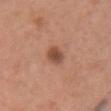A lesion tile, about 15 mm wide, cut from a 3D total-body photograph.
A female subject aged 33 to 37.
Imaged with white-light lighting.
The recorded lesion diameter is about 2.5 mm.
The lesion is on the front of the torso.